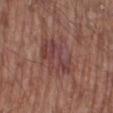Recorded during total-body skin imaging; not selected for excision or biopsy. The tile uses white-light illumination. A 15 mm crop from a total-body photograph taken for skin-cancer surveillance. Measured at roughly 5.5 mm in maximum diameter. The lesion is on the right lower leg. Automated image analysis of the tile measured a border-irregularity index near 5.5/10, internal color variation of about 5 on a 0–10 scale, and a peripheral color-asymmetry measure near 1.5. The analysis additionally found a nevus-likeness score of about 0/100. A male patient, approximately 80 years of age.